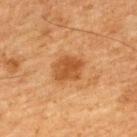{
  "biopsy_status": "not biopsied; imaged during a skin examination",
  "image": {
    "source": "total-body photography crop",
    "field_of_view_mm": 15
  },
  "patient": {
    "sex": "male",
    "age_approx": 65
  },
  "site": "upper back",
  "lesion_size": {
    "long_diameter_mm_approx": 4.0
  },
  "lighting": "cross-polarized"
}